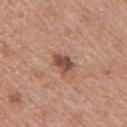notes — imaged on a skin check; not biopsied | acquisition — ~15 mm tile from a whole-body skin photo | patient — female, roughly 70 years of age | tile lighting — white-light | body site — the right upper arm | lesion diameter — ≈3 mm.